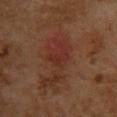Assessment:
This lesion was catalogued during total-body skin photography and was not selected for biopsy.
Acquisition and patient details:
The subject is a female aged 58–62. This image is a 15 mm lesion crop taken from a total-body photograph. Located on the upper back. This is a cross-polarized tile. About 3 mm across.